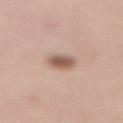Impression: Recorded during total-body skin imaging; not selected for excision or biopsy. Context: Approximately 3.5 mm at its widest. The lesion is on the mid back. A close-up tile cropped from a whole-body skin photograph, about 15 mm across. A female subject aged 48–52. The tile uses white-light illumination.